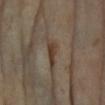Clinical impression:
The lesion was photographed on a routine skin check and not biopsied; there is no pathology result.
Background:
On the left forearm. The patient is a female in their mid- to late 70s. Longest diameter approximately 3 mm. Imaged with cross-polarized lighting. A region of skin cropped from a whole-body photographic capture, roughly 15 mm wide.